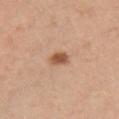| field | value |
|---|---|
| notes | catalogued during a skin exam; not biopsied |
| image source | ~15 mm tile from a whole-body skin photo |
| anatomic site | the chest |
| subject | female, aged approximately 45 |